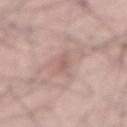biopsy status = imaged on a skin check; not biopsied
lesion diameter = ~3 mm (longest diameter)
site = the abdomen
subject = male, roughly 65 years of age
image = ~15 mm tile from a whole-body skin photo
illumination = white-light illumination